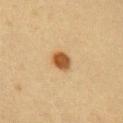Captured during whole-body skin photography for melanoma surveillance; the lesion was not biopsied.
The lesion-visualizer software estimated an outline eccentricity of about 0.55 (0 = round, 1 = elongated) and two-axis asymmetry of about 0.2. The analysis additionally found internal color variation of about 3.5 on a 0–10 scale and radial color variation of about 1. And it measured a classifier nevus-likeness of about 100/100 and lesion-presence confidence of about 100/100.
On the right upper arm.
Captured under cross-polarized illumination.
A close-up tile cropped from a whole-body skin photograph, about 15 mm across.
A female patient, about 30 years old.